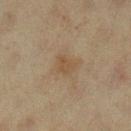The lesion was photographed on a routine skin check and not biopsied; there is no pathology result. The lesion is located on the leg. The lesion's longest dimension is about 2.5 mm. A close-up tile cropped from a whole-body skin photograph, about 15 mm across. A female subject, approximately 40 years of age. This is a cross-polarized tile.